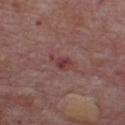Findings:
• biopsy status — no biopsy performed (imaged during a skin exam)
• anatomic site — the chest
• acquisition — total-body-photography crop, ~15 mm field of view
• automated lesion analysis — a lesion color around L≈39 a*≈25 b*≈19 in CIELAB, about 8 CIELAB-L* units darker than the surrounding skin, and a normalized border contrast of about 7; a detector confidence of about 100 out of 100 that the crop contains a lesion
• subject — male, about 75 years old